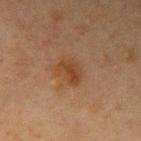notes: total-body-photography surveillance lesion; no biopsy | lighting: cross-polarized illumination | subject: male, in their mid- to late 60s | image source: 15 mm crop, total-body photography | location: the arm | image-analysis metrics: border irregularity of about 3 on a 0–10 scale, a within-lesion color-variation index near 3/10, and radial color variation of about 1 | diameter: about 3 mm.